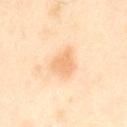<record>
  <biopsy_status>not biopsied; imaged during a skin examination</biopsy_status>
  <lighting>cross-polarized</lighting>
  <site>left thigh</site>
  <automated_metrics>
    <cielab_L>75</cielab_L>
    <cielab_a>20</cielab_a>
    <cielab_b>40</cielab_b>
    <vs_skin_darker_L>8.0</vs_skin_darker_L>
    <vs_skin_contrast_norm>5.5</vs_skin_contrast_norm>
    <border_irregularity_0_10>3.5</border_irregularity_0_10>
    <peripheral_color_asymmetry>0.5</peripheral_color_asymmetry>
  </automated_metrics>
  <patient>
    <sex>male</sex>
    <age_approx>55</age_approx>
  </patient>
  <lesion_size>
    <long_diameter_mm_approx>3.0</long_diameter_mm_approx>
  </lesion_size>
  <image>
    <source>total-body photography crop</source>
    <field_of_view_mm>15</field_of_view_mm>
  </image>
</record>